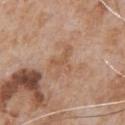Recorded during total-body skin imaging; not selected for excision or biopsy.
This is a white-light tile.
From the chest.
Automated tile analysis of the lesion measured an area of roughly 6 mm² and an eccentricity of roughly 0.75. The analysis additionally found a color-variation rating of about 1/10 and peripheral color asymmetry of about 0.5.
The patient is a male roughly 65 years of age.
A 15 mm crop from a total-body photograph taken for skin-cancer surveillance.